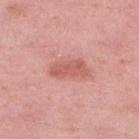Impression: The lesion was photographed on a routine skin check and not biopsied; there is no pathology result.